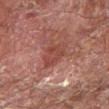Findings:
- site: the right forearm
- image: ~15 mm crop, total-body skin-cancer survey
- patient: male, approximately 70 years of age